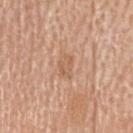A close-up tile cropped from a whole-body skin photograph, about 15 mm across.
The patient is a female aged around 70.
On the head or neck.
The recorded lesion diameter is about 2.5 mm.
The tile uses white-light illumination.
An algorithmic analysis of the crop reported an area of roughly 3.5 mm², a shape eccentricity near 0.75, and a symmetry-axis asymmetry near 0.3. It also reported a lesion color around L≈59 a*≈21 b*≈33 in CIELAB, about 7 CIELAB-L* units darker than the surrounding skin, and a normalized border contrast of about 5. The software also gave a nevus-likeness score of about 0/100 and a detector confidence of about 100 out of 100 that the crop contains a lesion.